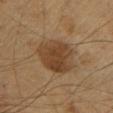Clinical impression: Recorded during total-body skin imaging; not selected for excision or biopsy. Image and clinical context: The subject is a male aged around 60. The lesion-visualizer software estimated an area of roughly 18 mm², an outline eccentricity of about 0.4 (0 = round, 1 = elongated), and a symmetry-axis asymmetry near 0.15. The software also gave a mean CIELAB color near L≈37 a*≈15 b*≈29, a lesion–skin lightness drop of about 9, and a lesion-to-skin contrast of about 8 (normalized; higher = more distinct). The lesion is located on the upper back. Captured under cross-polarized illumination. The recorded lesion diameter is about 5 mm. A 15 mm crop from a total-body photograph taken for skin-cancer surveillance.